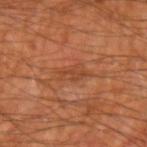Captured during whole-body skin photography for melanoma surveillance; the lesion was not biopsied. The lesion is on the left upper arm. A male subject, aged 58–62. About 3 mm across. A 15 mm crop from a total-body photograph taken for skin-cancer surveillance.